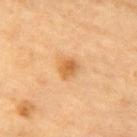  biopsy_status: not biopsied; imaged during a skin examination
  patient:
    sex: male
    age_approx: 85+
  lesion_size:
    long_diameter_mm_approx: 2.5
  image:
    source: total-body photography crop
    field_of_view_mm: 15
  site: chest